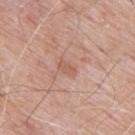biopsy status: total-body-photography surveillance lesion; no biopsy | site: the mid back | image source: ~15 mm crop, total-body skin-cancer survey | subject: male, aged around 65 | lesion size: ~3 mm (longest diameter) | image-analysis metrics: an average lesion color of about L≈59 a*≈22 b*≈28 (CIELAB), a lesion–skin lightness drop of about 7, and a normalized border contrast of about 4.5; a nevus-likeness score of about 0/100 and lesion-presence confidence of about 100/100.